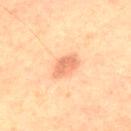{"biopsy_status": "not biopsied; imaged during a skin examination", "site": "chest", "patient": {"sex": "male", "age_approx": 65}, "lesion_size": {"long_diameter_mm_approx": 3.5}, "image": {"source": "total-body photography crop", "field_of_view_mm": 15}, "lighting": "cross-polarized"}